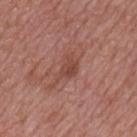Captured during whole-body skin photography for melanoma surveillance; the lesion was not biopsied. On the mid back. The patient is a male roughly 65 years of age. Captured under white-light illumination. Longest diameter approximately 3.5 mm. A lesion tile, about 15 mm wide, cut from a 3D total-body photograph. Automated tile analysis of the lesion measured a lesion area of about 5.5 mm², an eccentricity of roughly 0.8, and a symmetry-axis asymmetry near 0.45. And it measured a nevus-likeness score of about 0/100 and lesion-presence confidence of about 100/100.